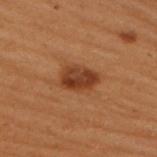The lesion was photographed on a routine skin check and not biopsied; there is no pathology result.
This is a cross-polarized tile.
Longest diameter approximately 4 mm.
On the back.
A female subject, aged approximately 50.
This image is a 15 mm lesion crop taken from a total-body photograph.